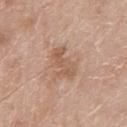follow-up: catalogued during a skin exam; not biopsied | site: the front of the torso | automated lesion analysis: an area of roughly 7.5 mm², a shape eccentricity near 0.8, and a shape-asymmetry score of about 0.4 (0 = symmetric); roughly 8 lightness units darker than nearby skin and a lesion-to-skin contrast of about 5.5 (normalized; higher = more distinct); a border-irregularity index near 5.5/10 and a peripheral color-asymmetry measure near 1; a classifier nevus-likeness of about 0/100 and a detector confidence of about 100 out of 100 that the crop contains a lesion | image source: 15 mm crop, total-body photography | lesion size: ≈4 mm | lighting: white-light illumination | patient: male, approximately 80 years of age.